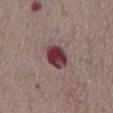Q: Was a biopsy performed?
A: no biopsy performed (imaged during a skin exam)
Q: What did automated image analysis measure?
A: a lesion color around L≈37 a*≈26 b*≈14 in CIELAB, roughly 17 lightness units darker than nearby skin, and a lesion-to-skin contrast of about 14 (normalized; higher = more distinct)
Q: What kind of image is this?
A: ~15 mm crop, total-body skin-cancer survey
Q: Where on the body is the lesion?
A: the chest
Q: Lesion size?
A: ≈3.5 mm
Q: What lighting was used for the tile?
A: white-light illumination
Q: Patient demographics?
A: male, aged approximately 75